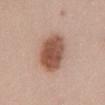Case summary:
- lighting · white-light
- anatomic site · the upper back
- image source · ~15 mm tile from a whole-body skin photo
- lesion diameter · ≈7 mm
- patient · male, roughly 55 years of age